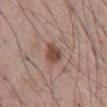{
  "lesion_size": {
    "long_diameter_mm_approx": 3.0
  },
  "image": {
    "source": "total-body photography crop",
    "field_of_view_mm": 15
  },
  "automated_metrics": {
    "vs_skin_darker_L": 12.0,
    "vs_skin_contrast_norm": 9.0,
    "color_variation_0_10": 3.0,
    "peripheral_color_asymmetry": 1.0
  },
  "site": "abdomen",
  "patient": {
    "sex": "male",
    "age_approx": 55
  }
}